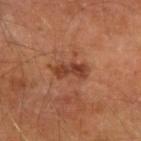notes: imaged on a skin check; not biopsied | acquisition: ~15 mm tile from a whole-body skin photo | TBP lesion metrics: a lesion area of about 6 mm², an eccentricity of roughly 0.9, and a shape-asymmetry score of about 0.35 (0 = symmetric); border irregularity of about 4 on a 0–10 scale, internal color variation of about 3.5 on a 0–10 scale, and a peripheral color-asymmetry measure near 1 | illumination: cross-polarized | patient: male, in their mid- to late 60s | anatomic site: the right upper arm | lesion size: ≈4 mm.